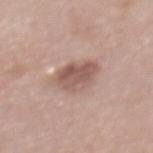Clinical impression: Imaged during a routine full-body skin examination; the lesion was not biopsied and no histopathology is available.